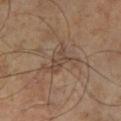| field | value |
|---|---|
| patient | male, aged 58 to 62 |
| acquisition | ~15 mm crop, total-body skin-cancer survey |
| location | the leg |